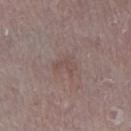- follow-up · no biopsy performed (imaged during a skin exam)
- image-analysis metrics · an outline eccentricity of about 0.85 (0 = round, 1 = elongated); border irregularity of about 6 on a 0–10 scale and a within-lesion color-variation index near 0/10
- lighting · white-light illumination
- lesion size · ≈2.5 mm
- anatomic site · the right lower leg
- image · ~15 mm tile from a whole-body skin photo
- patient · male, aged 73–77